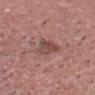This lesion was catalogued during total-body skin photography and was not selected for biopsy.
The lesion's longest dimension is about 3.5 mm.
A male subject in their mid-50s.
Imaged with white-light lighting.
Automated tile analysis of the lesion measured an average lesion color of about L≈47 a*≈21 b*≈23 (CIELAB) and about 9 CIELAB-L* units darker than the surrounding skin. The software also gave border irregularity of about 3.5 on a 0–10 scale, a color-variation rating of about 4/10, and a peripheral color-asymmetry measure near 1.5.
A roughly 15 mm field-of-view crop from a total-body skin photograph.
From the head or neck.